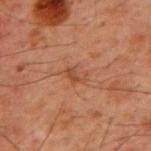follow-up: catalogued during a skin exam; not biopsied | imaging modality: ~15 mm crop, total-body skin-cancer survey | diameter: ~2.5 mm (longest diameter) | site: the back | subject: male, in their 60s | illumination: cross-polarized.